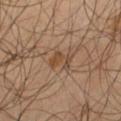Q: Was this lesion biopsied?
A: catalogued during a skin exam; not biopsied
Q: What is the anatomic site?
A: the left thigh
Q: How large is the lesion?
A: ~3 mm (longest diameter)
Q: How was the tile lit?
A: cross-polarized
Q: Automated lesion metrics?
A: a footprint of about 4.5 mm² and an eccentricity of roughly 0.7; an average lesion color of about L≈41 a*≈16 b*≈29 (CIELAB), a lesion–skin lightness drop of about 7, and a lesion-to-skin contrast of about 6.5 (normalized; higher = more distinct)
Q: What kind of image is this?
A: total-body-photography crop, ~15 mm field of view
Q: What are the patient's age and sex?
A: male, about 55 years old